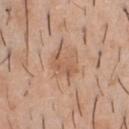No biopsy was performed on this lesion — it was imaged during a full skin examination and was not determined to be concerning.
From the chest.
Cropped from a total-body skin-imaging series; the visible field is about 15 mm.
Automated tile analysis of the lesion measured a footprint of about 8 mm² and an eccentricity of roughly 0.5. And it measured a mean CIELAB color near L≈58 a*≈20 b*≈32 and about 8 CIELAB-L* units darker than the surrounding skin. And it measured a border-irregularity index near 3.5/10, a within-lesion color-variation index near 2.5/10, and a peripheral color-asymmetry measure near 1.
Approximately 3.5 mm at its widest.
Imaged with white-light lighting.
The subject is a male roughly 40 years of age.